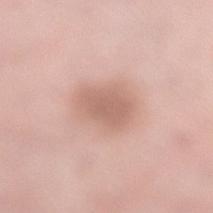follow-up: catalogued during a skin exam; not biopsied | body site: the left lower leg | tile lighting: white-light illumination | subject: female, aged 38–42 | TBP lesion metrics: an area of roughly 8.5 mm², a shape eccentricity near 0.7, and two-axis asymmetry of about 0.2; roughly 10 lightness units darker than nearby skin and a lesion-to-skin contrast of about 6.5 (normalized; higher = more distinct); internal color variation of about 1.5 on a 0–10 scale and peripheral color asymmetry of about 0.5; an automated nevus-likeness rating near 55 out of 100 | imaging modality: 15 mm crop, total-body photography.